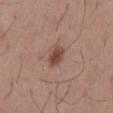Impression: The lesion was tiled from a total-body skin photograph and was not biopsied.